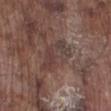No biopsy was performed on this lesion — it was imaged during a full skin examination and was not determined to be concerning. The subject is a male aged approximately 75. Longest diameter approximately 5.5 mm. Imaged with white-light lighting. A 15 mm close-up extracted from a 3D total-body photography capture. The lesion is located on the right lower leg. An algorithmic analysis of the crop reported roughly 7 lightness units darker than nearby skin.